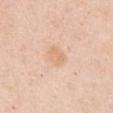<case>
  <biopsy_status>not biopsied; imaged during a skin examination</biopsy_status>
  <patient>
    <sex>female</sex>
    <age_approx>50</age_approx>
  </patient>
  <site>chest</site>
  <automated_metrics>
    <area_mm2_approx>5.0</area_mm2_approx>
    <eccentricity>0.65</eccentricity>
    <shape_asymmetry>0.2</shape_asymmetry>
    <cielab_L>72</cielab_L>
    <cielab_a>19</cielab_a>
    <cielab_b>34</cielab_b>
    <vs_skin_contrast_norm>5.0</vs_skin_contrast_norm>
    <nevus_likeness_0_100>0</nevus_likeness_0_100>
    <lesion_detection_confidence_0_100>100</lesion_detection_confidence_0_100>
  </automated_metrics>
  <image>
    <source>total-body photography crop</source>
    <field_of_view_mm>15</field_of_view_mm>
  </image>
  <lesion_size>
    <long_diameter_mm_approx>3.0</long_diameter_mm_approx>
  </lesion_size>
  <lighting>white-light</lighting>
</case>